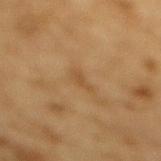No biopsy was performed on this lesion — it was imaged during a full skin examination and was not determined to be concerning.
Located on the mid back.
Cropped from a total-body skin-imaging series; the visible field is about 15 mm.
The recorded lesion diameter is about 2.5 mm.
The subject is a male in their mid- to late 80s or older.
Automated tile analysis of the lesion measured a lesion area of about 2.5 mm², a shape eccentricity near 0.9, and a symmetry-axis asymmetry near 0.4. The software also gave an average lesion color of about L≈43 a*≈16 b*≈33 (CIELAB), roughly 6 lightness units darker than nearby skin, and a normalized lesion–skin contrast near 5. It also reported a nevus-likeness score of about 0/100 and lesion-presence confidence of about 100/100.
Captured under cross-polarized illumination.